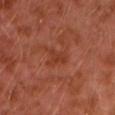Findings:
– follow-up: no biopsy performed (imaged during a skin exam)
– body site: the left upper arm
– acquisition: total-body-photography crop, ~15 mm field of view
– illumination: cross-polarized
– size: about 2.5 mm
– subject: male, aged 28–32